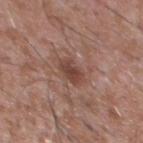Image and clinical context: The recorded lesion diameter is about 3 mm. A male subject aged approximately 45. A 15 mm close-up tile from a total-body photography series done for melanoma screening. The tile uses white-light illumination. Located on the chest.